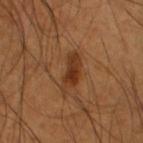biopsy status = catalogued during a skin exam; not biopsied | site = the left lower leg | diameter = about 4.5 mm | imaging modality = ~15 mm tile from a whole-body skin photo | patient = male, aged approximately 55 | tile lighting = cross-polarized | image-analysis metrics = a footprint of about 7 mm², an eccentricity of roughly 0.9, and a symmetry-axis asymmetry near 0.4; a peripheral color-asymmetry measure near 1; an automated nevus-likeness rating near 95 out of 100 and a detector confidence of about 100 out of 100 that the crop contains a lesion.